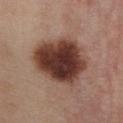follow-up: total-body-photography surveillance lesion; no biopsy | diameter: about 7 mm | acquisition: total-body-photography crop, ~15 mm field of view | site: the chest | tile lighting: white-light | subject: female, in their mid- to late 50s.